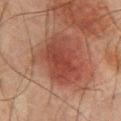Q: Is there a histopathology result?
A: no biopsy performed (imaged during a skin exam)
Q: Where on the body is the lesion?
A: the back
Q: How large is the lesion?
A: ≈5.5 mm
Q: What kind of image is this?
A: 15 mm crop, total-body photography
Q: What lighting was used for the tile?
A: cross-polarized illumination
Q: What did automated image analysis measure?
A: a footprint of about 16 mm², an outline eccentricity of about 0.75 (0 = round, 1 = elongated), and a symmetry-axis asymmetry near 0.25
Q: Patient demographics?
A: male, roughly 65 years of age